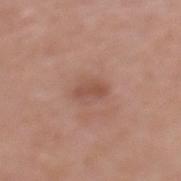Part of a total-body skin-imaging series; this lesion was reviewed on a skin check and was not flagged for biopsy. The lesion is located on the back. The patient is a female about 70 years old. A lesion tile, about 15 mm wide, cut from a 3D total-body photograph.